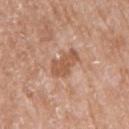workup = catalogued during a skin exam; not biopsied | subject = female, aged approximately 75 | lesion size = ≈4 mm | lighting = white-light illumination | image = ~15 mm tile from a whole-body skin photo | location = the arm | automated lesion analysis = a lesion color around L≈55 a*≈22 b*≈32 in CIELAB and a normalized lesion–skin contrast near 7; a border-irregularity rating of about 3.5/10 and radial color variation of about 0.5.